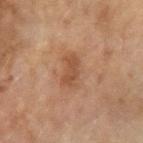Q: Is there a histopathology result?
A: catalogued during a skin exam; not biopsied
Q: Patient demographics?
A: female, in their 60s
Q: How was this image acquired?
A: ~15 mm crop, total-body skin-cancer survey
Q: What is the lesion's diameter?
A: ~3.5 mm (longest diameter)
Q: What lighting was used for the tile?
A: cross-polarized illumination
Q: Automated lesion metrics?
A: an outline eccentricity of about 0.9 (0 = round, 1 = elongated) and a shape-asymmetry score of about 0.25 (0 = symmetric); roughly 8 lightness units darker than nearby skin and a normalized lesion–skin contrast near 6.5
Q: Where on the body is the lesion?
A: the left forearm